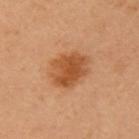Case summary:
- biopsy status: total-body-photography surveillance lesion; no biopsy
- lesion diameter: ~5 mm (longest diameter)
- imaging modality: 15 mm crop, total-body photography
- tile lighting: cross-polarized
- site: the chest
- subject: female, in their 60s
- image-analysis metrics: a lesion–skin lightness drop of about 10 and a normalized lesion–skin contrast near 8; border irregularity of about 2 on a 0–10 scale, a color-variation rating of about 4/10, and peripheral color asymmetry of about 1.5; an automated nevus-likeness rating near 100 out of 100 and a detector confidence of about 100 out of 100 that the crop contains a lesion